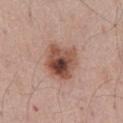  biopsy_status: not biopsied; imaged during a skin examination
  lesion_size:
    long_diameter_mm_approx: 4.5
  lighting: white-light
  patient:
    sex: male
    age_approx: 55
  image:
    source: total-body photography crop
    field_of_view_mm: 15
  site: abdomen